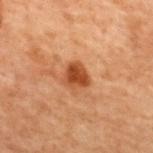Recorded during total-body skin imaging; not selected for excision or biopsy.
The lesion is on the mid back.
The patient is a male aged 68–72.
This image is a 15 mm lesion crop taken from a total-body photograph.
Approximately 3 mm at its widest.